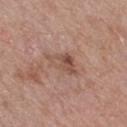Notes:
* notes — no biopsy performed (imaged during a skin exam)
* image source — ~15 mm crop, total-body skin-cancer survey
* location — the right forearm
* lesion diameter — about 4.5 mm
* illumination — white-light illumination
* automated metrics — a footprint of about 7 mm², a shape eccentricity near 0.85, and two-axis asymmetry of about 0.35; a classifier nevus-likeness of about 0/100 and a detector confidence of about 100 out of 100 that the crop contains a lesion
* patient — female, aged 48–52